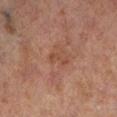Recorded during total-body skin imaging; not selected for excision or biopsy.
Cropped from a whole-body photographic skin survey; the tile spans about 15 mm.
A male subject in their 70s.
Captured under cross-polarized illumination.
On the leg.